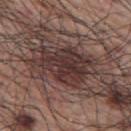Captured during whole-body skin photography for melanoma surveillance; the lesion was not biopsied. A male subject aged 68 to 72. Imaged with white-light lighting. Located on the upper back. The lesion's longest dimension is about 6 mm. Automated image analysis of the tile measured an area of roughly 18 mm², an eccentricity of roughly 0.7, and a symmetry-axis asymmetry near 0.25. It also reported a lesion color around L≈33 a*≈17 b*≈18 in CIELAB and a normalized border contrast of about 10. The software also gave border irregularity of about 3 on a 0–10 scale and a color-variation rating of about 4.5/10. The analysis additionally found an automated nevus-likeness rating near 30 out of 100 and a lesion-detection confidence of about 100/100. A 15 mm close-up extracted from a 3D total-body photography capture.